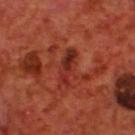Q: Was a biopsy performed?
A: no biopsy performed (imaged during a skin exam)
Q: What are the patient's age and sex?
A: male, aged around 70
Q: Where on the body is the lesion?
A: the back
Q: What is the imaging modality?
A: 15 mm crop, total-body photography